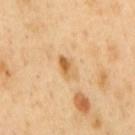The lesion was tiled from a total-body skin photograph and was not biopsied. Located on the mid back. A close-up tile cropped from a whole-body skin photograph, about 15 mm across. The lesion's longest dimension is about 3 mm. The patient is a male in their 50s.